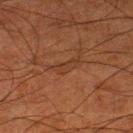The lesion was photographed on a routine skin check and not biopsied; there is no pathology result. The lesion is located on the left lower leg. A lesion tile, about 15 mm wide, cut from a 3D total-body photograph. A male patient about 80 years old.